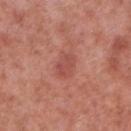No biopsy was performed on this lesion — it was imaged during a full skin examination and was not determined to be concerning.
The tile uses white-light illumination.
Automated tile analysis of the lesion measured a footprint of about 5.5 mm² and an eccentricity of roughly 0.75.
A male subject, aged 53–57.
The lesion's longest dimension is about 3 mm.
Located on the leg.
A roughly 15 mm field-of-view crop from a total-body skin photograph.